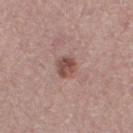{"biopsy_status": "not biopsied; imaged during a skin examination", "patient": {"sex": "female", "age_approx": 40}, "image": {"source": "total-body photography crop", "field_of_view_mm": 15}, "lesion_size": {"long_diameter_mm_approx": 2.5}, "lighting": "white-light", "site": "right thigh"}